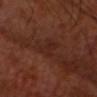Assessment: Imaged during a routine full-body skin examination; the lesion was not biopsied and no histopathology is available. Context: Imaged with cross-polarized lighting. The patient is a male approximately 60 years of age. The lesion is on the head or neck. A 15 mm close-up tile from a total-body photography series done for melanoma screening.